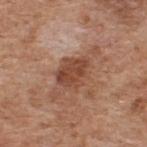anatomic site = the upper back | TBP lesion metrics = an area of roughly 14 mm², an eccentricity of roughly 0.9, and a symmetry-axis asymmetry near 0.25; an average lesion color of about L≈47 a*≈22 b*≈30 (CIELAB), about 10 CIELAB-L* units darker than the surrounding skin, and a normalized lesion–skin contrast near 8; border irregularity of about 5 on a 0–10 scale, a within-lesion color-variation index near 3.5/10, and radial color variation of about 1 | lighting = white-light | subject = male, aged 58 to 62 | diameter = ≈7 mm | image source = ~15 mm tile from a whole-body skin photo.